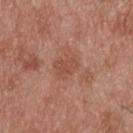{
  "site": "upper back",
  "lighting": "white-light",
  "patient": {
    "sex": "male",
    "age_approx": 55
  },
  "image": {
    "source": "total-body photography crop",
    "field_of_view_mm": 15
  }
}